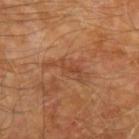The lesion was tiled from a total-body skin photograph and was not biopsied. On the left upper arm. This is a cross-polarized tile. A male patient, about 70 years old. A lesion tile, about 15 mm wide, cut from a 3D total-body photograph. About 5.5 mm across.